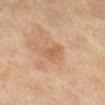Captured during whole-body skin photography for melanoma surveillance; the lesion was not biopsied. On the right lower leg. A close-up tile cropped from a whole-body skin photograph, about 15 mm across. Automated image analysis of the tile measured an area of roughly 3.5 mm², a shape eccentricity near 0.85, and a symmetry-axis asymmetry near 0.35. Longest diameter approximately 2.5 mm. The subject is a female in their 40s.